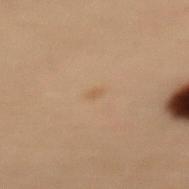Assessment:
This lesion was catalogued during total-body skin photography and was not selected for biopsy.
Context:
Captured under cross-polarized illumination. About 1 mm across. A female subject in their 50s. Automated image analysis of the tile measured a lesion–skin lightness drop of about 5 and a normalized lesion–skin contrast near 5. Located on the mid back. Cropped from a total-body skin-imaging series; the visible field is about 15 mm.